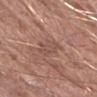Assessment:
The lesion was tiled from a total-body skin photograph and was not biopsied.
Context:
Approximately 3.5 mm at its widest. From the arm. A region of skin cropped from a whole-body photographic capture, roughly 15 mm wide. Automated image analysis of the tile measured an area of roughly 6 mm² and an eccentricity of roughly 0.85. And it measured an average lesion color of about L≈50 a*≈19 b*≈24 (CIELAB). A male patient in their mid-60s.